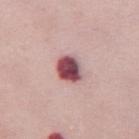Q: Was a biopsy performed?
A: imaged on a skin check; not biopsied
Q: What are the patient's age and sex?
A: female, in their mid- to late 60s
Q: What lighting was used for the tile?
A: white-light illumination
Q: What is the lesion's diameter?
A: ≈3 mm
Q: What is the anatomic site?
A: the chest
Q: What kind of image is this?
A: ~15 mm tile from a whole-body skin photo